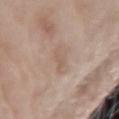Assessment:
Recorded during total-body skin imaging; not selected for excision or biopsy.
Image and clinical context:
Captured under white-light illumination. An algorithmic analysis of the crop reported a footprint of about 4 mm², an outline eccentricity of about 0.95 (0 = round, 1 = elongated), and two-axis asymmetry of about 0.3. It also reported an average lesion color of about L≈58 a*≈16 b*≈27 (CIELAB), about 6 CIELAB-L* units darker than the surrounding skin, and a lesion-to-skin contrast of about 4.5 (normalized; higher = more distinct). And it measured lesion-presence confidence of about 100/100. A female subject, aged around 75. Located on the right forearm. The lesion's longest dimension is about 3.5 mm. Cropped from a total-body skin-imaging series; the visible field is about 15 mm.